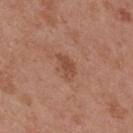Assessment:
Recorded during total-body skin imaging; not selected for excision or biopsy.
Context:
A 15 mm close-up tile from a total-body photography series done for melanoma screening. Longest diameter approximately 3 mm. A female subject, in their 40s. The lesion-visualizer software estimated a border-irregularity index near 3.5/10 and a within-lesion color-variation index near 1/10. And it measured a nevus-likeness score of about 20/100 and lesion-presence confidence of about 100/100. On the upper back.